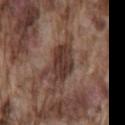{
  "biopsy_status": "not biopsied; imaged during a skin examination",
  "image": {
    "source": "total-body photography crop",
    "field_of_view_mm": 15
  },
  "lesion_size": {
    "long_diameter_mm_approx": 5.0
  },
  "patient": {
    "sex": "male",
    "age_approx": 75
  },
  "site": "lower back"
}